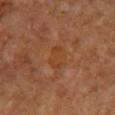The lesion was tiled from a total-body skin photograph and was not biopsied. An algorithmic analysis of the crop reported a border-irregularity index near 4/10, a color-variation rating of about 1.5/10, and radial color variation of about 0.5. The recorded lesion diameter is about 3 mm. Imaged with cross-polarized lighting. Cropped from a whole-body photographic skin survey; the tile spans about 15 mm. A female subject, approximately 70 years of age. Located on the right upper arm.